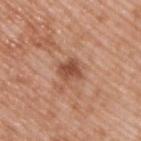Imaged during a routine full-body skin examination; the lesion was not biopsied and no histopathology is available. The tile uses white-light illumination. A region of skin cropped from a whole-body photographic capture, roughly 15 mm wide. Located on the upper back. Measured at roughly 3 mm in maximum diameter. An algorithmic analysis of the crop reported an area of roughly 5.5 mm², an outline eccentricity of about 0.55 (0 = round, 1 = elongated), and a shape-asymmetry score of about 0.25 (0 = symmetric). The software also gave a nevus-likeness score of about 15/100 and lesion-presence confidence of about 100/100. A male subject in their 50s.